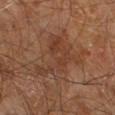Impression: Recorded during total-body skin imaging; not selected for excision or biopsy. Context: A 15 mm close-up extracted from a 3D total-body photography capture. Captured under cross-polarized illumination. The lesion's longest dimension is about 7 mm. From the right leg. The subject is a male aged 58 to 62.